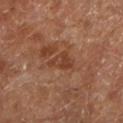{
  "biopsy_status": "not biopsied; imaged during a skin examination"
}